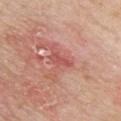Clinical impression:
No biopsy was performed on this lesion — it was imaged during a full skin examination and was not determined to be concerning.
Acquisition and patient details:
The lesion is located on the chest. Captured under white-light illumination. A region of skin cropped from a whole-body photographic capture, roughly 15 mm wide. The subject is a female about 70 years old.